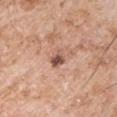| field | value |
|---|---|
| biopsy status | imaged on a skin check; not biopsied |
| imaging modality | ~15 mm crop, total-body skin-cancer survey |
| lighting | white-light |
| subject | male, in their mid- to late 50s |
| body site | the right upper arm |